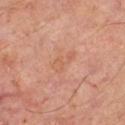{"biopsy_status": "not biopsied; imaged during a skin examination", "automated_metrics": {"area_mm2_approx": 3.5, "eccentricity": 0.9, "shape_asymmetry": 0.5, "cielab_L": 57, "cielab_a": 23, "cielab_b": 33, "vs_skin_contrast_norm": 4.5}, "lighting": "cross-polarized", "patient": {"sex": "male", "age_approx": 70}, "site": "left thigh", "image": {"source": "total-body photography crop", "field_of_view_mm": 15}}